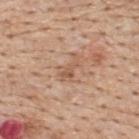Part of a total-body skin-imaging series; this lesion was reviewed on a skin check and was not flagged for biopsy. Measured at roughly 3 mm in maximum diameter. This is a white-light tile. A male subject, in their 60s. The lesion is on the upper back. Cropped from a whole-body photographic skin survey; the tile spans about 15 mm.